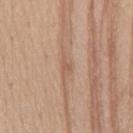biopsy_status: not biopsied; imaged during a skin examination
lighting: white-light
automated_metrics:
  area_mm2_approx: 2.5
  eccentricity: 0.9
  cielab_L: 58
  cielab_a: 19
  cielab_b: 30
  border_irregularity_0_10: 3.5
  color_variation_0_10: 1.0
  peripheral_color_asymmetry: 0.0
  lesion_detection_confidence_0_100: 75
site: mid back
image:
  source: total-body photography crop
  field_of_view_mm: 15
patient:
  sex: female
  age_approx: 50